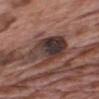Findings:
• biopsy status: catalogued during a skin exam; not biopsied
• illumination: white-light
• body site: the upper back
• lesion size: ~5.5 mm (longest diameter)
• image: ~15 mm tile from a whole-body skin photo
• patient: male, about 70 years old
• automated lesion analysis: an area of roughly 18 mm², an outline eccentricity of about 0.7 (0 = round, 1 = elongated), and two-axis asymmetry of about 0.3